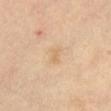Imaged during a routine full-body skin examination; the lesion was not biopsied and no histopathology is available. On the abdomen. A close-up tile cropped from a whole-body skin photograph, about 15 mm across. Approximately 2.5 mm at its widest. A female patient, about 60 years old. Imaged with cross-polarized lighting. Automated tile analysis of the lesion measured an area of roughly 3 mm², a shape eccentricity near 0.9, and a shape-asymmetry score of about 0.3 (0 = symmetric). And it measured a mean CIELAB color near L≈70 a*≈16 b*≈38, a lesion–skin lightness drop of about 6, and a lesion-to-skin contrast of about 4.5 (normalized; higher = more distinct). It also reported a classifier nevus-likeness of about 0/100 and a detector confidence of about 100 out of 100 that the crop contains a lesion.